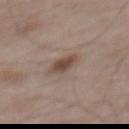biopsy_status: not biopsied; imaged during a skin examination
lighting: white-light
patient:
  sex: male
  age_approx: 55
image:
  source: total-body photography crop
  field_of_view_mm: 15
site: back
automated_metrics:
  area_mm2_approx: 5.0
  eccentricity: 0.85
  shape_asymmetry: 0.2
  border_irregularity_0_10: 2.0
  color_variation_0_10: 2.0
  peripheral_color_asymmetry: 0.5
  nevus_likeness_0_100: 95
  lesion_detection_confidence_0_100: 100
lesion_size:
  long_diameter_mm_approx: 3.5